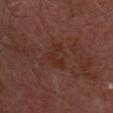Q: Is there a histopathology result?
A: total-body-photography surveillance lesion; no biopsy
Q: Lesion location?
A: the head or neck
Q: What are the patient's age and sex?
A: male, aged approximately 60
Q: Illumination type?
A: cross-polarized
Q: What kind of image is this?
A: total-body-photography crop, ~15 mm field of view
Q: Lesion size?
A: ≈3 mm
Q: What did automated image analysis measure?
A: a mean CIELAB color near L≈27 a*≈21 b*≈24 and a lesion-to-skin contrast of about 6 (normalized; higher = more distinct); a border-irregularity index near 8/10 and a peripheral color-asymmetry measure near 0; a nevus-likeness score of about 0/100 and lesion-presence confidence of about 100/100